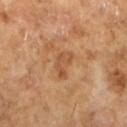Imaged during a routine full-body skin examination; the lesion was not biopsied and no histopathology is available. A male subject in their mid- to late 60s. A roughly 15 mm field-of-view crop from a total-body skin photograph.